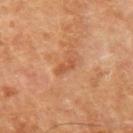The lesion was tiled from a total-body skin photograph and was not biopsied. A close-up tile cropped from a whole-body skin photograph, about 15 mm across. A male subject, aged 68–72. On the left upper arm. Automated tile analysis of the lesion measured an average lesion color of about L≈53 a*≈27 b*≈37 (CIELAB), about 8 CIELAB-L* units darker than the surrounding skin, and a normalized border contrast of about 6. It also reported border irregularity of about 6 on a 0–10 scale, a within-lesion color-variation index near 0/10, and radial color variation of about 0. The software also gave a nevus-likeness score of about 0/100 and lesion-presence confidence of about 100/100. Measured at roughly 3 mm in maximum diameter.